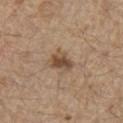Part of a total-body skin-imaging series; this lesion was reviewed on a skin check and was not flagged for biopsy.
About 3 mm across.
A 15 mm close-up extracted from a 3D total-body photography capture.
The tile uses white-light illumination.
The patient is a male roughly 70 years of age.
Automated image analysis of the tile measured a lesion area of about 5 mm² and an eccentricity of roughly 0.7. The software also gave a lesion color around L≈48 a*≈17 b*≈29 in CIELAB and a lesion–skin lightness drop of about 12.
The lesion is on the chest.